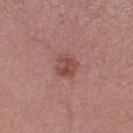biopsy status=total-body-photography surveillance lesion; no biopsy
anatomic site=the leg
tile lighting=white-light illumination
image=~15 mm tile from a whole-body skin photo
patient=female, approximately 75 years of age
size=≈3 mm
automated metrics=border irregularity of about 2.5 on a 0–10 scale, internal color variation of about 4 on a 0–10 scale, and a peripheral color-asymmetry measure near 1.5; a nevus-likeness score of about 65/100 and a detector confidence of about 100 out of 100 that the crop contains a lesion